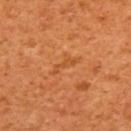diameter: ~3.5 mm (longest diameter)
anatomic site: the upper back
subject: female, in their mid-50s
tile lighting: cross-polarized
automated metrics: an area of roughly 2.5 mm² and an outline eccentricity of about 0.95 (0 = round, 1 = elongated); a normalized border contrast of about 5; a within-lesion color-variation index near 0/10 and a peripheral color-asymmetry measure near 0
image: ~15 mm crop, total-body skin-cancer survey